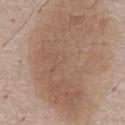Cropped from a whole-body photographic skin survey; the tile spans about 15 mm.
Longest diameter approximately 13 mm.
On the chest.
Imaged with white-light lighting.
An algorithmic analysis of the crop reported a border-irregularity rating of about 9/10 and internal color variation of about 3.5 on a 0–10 scale. The software also gave a nevus-likeness score of about 75/100 and lesion-presence confidence of about 95/100.
A male subject in their mid-50s.